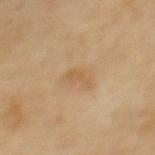The lesion was tiled from a total-body skin photograph and was not biopsied. The lesion's longest dimension is about 3 mm. This is a cross-polarized tile. On the upper back. A close-up tile cropped from a whole-body skin photograph, about 15 mm across. A female subject, about 70 years old. Automated tile analysis of the lesion measured an area of roughly 2.5 mm², a shape eccentricity near 0.9, and a symmetry-axis asymmetry near 0.55. It also reported a lesion color around L≈59 a*≈17 b*≈38 in CIELAB and a lesion-to-skin contrast of about 5.5 (normalized; higher = more distinct). It also reported a classifier nevus-likeness of about 5/100 and lesion-presence confidence of about 100/100.